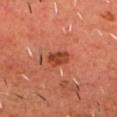{"biopsy_status": "not biopsied; imaged during a skin examination", "image": {"source": "total-body photography crop", "field_of_view_mm": 15}, "site": "head or neck", "patient": {"sex": "male", "age_approx": 55}}